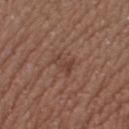Clinical impression: The lesion was tiled from a total-body skin photograph and was not biopsied. Acquisition and patient details: A 15 mm close-up tile from a total-body photography series done for melanoma screening. The lesion is located on the left lower leg. A female patient, aged approximately 40. Automated image analysis of the tile measured about 7 CIELAB-L* units darker than the surrounding skin. It also reported a nevus-likeness score of about 0/100 and lesion-presence confidence of about 100/100. The recorded lesion diameter is about 2.5 mm. Captured under white-light illumination.